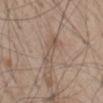follow-up: no biopsy performed (imaged during a skin exam) | patient: male, aged approximately 50 | acquisition: total-body-photography crop, ~15 mm field of view | site: the back | automated lesion analysis: a mean CIELAB color near L≈54 a*≈14 b*≈25 and a lesion-to-skin contrast of about 5 (normalized; higher = more distinct); a nevus-likeness score of about 0/100 and a detector confidence of about 75 out of 100 that the crop contains a lesion | lesion size: ~4.5 mm (longest diameter) | illumination: white-light illumination.